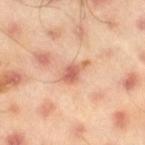Assessment:
The lesion was tiled from a total-body skin photograph and was not biopsied.
Acquisition and patient details:
On the right thigh. Measured at roughly 3.5 mm in maximum diameter. The subject is a male aged around 45. A 15 mm close-up tile from a total-body photography series done for melanoma screening. The tile uses cross-polarized illumination. An algorithmic analysis of the crop reported an area of roughly 5 mm², an outline eccentricity of about 0.85 (0 = round, 1 = elongated), and a symmetry-axis asymmetry near 0.4. The analysis additionally found lesion-presence confidence of about 100/100.